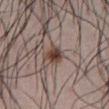workup: no biopsy performed (imaged during a skin exam)
patient: male, approximately 65 years of age
illumination: white-light
TBP lesion metrics: a footprint of about 4.5 mm² and a shape-asymmetry score of about 0.25 (0 = symmetric); an automated nevus-likeness rating near 95 out of 100 and a detector confidence of about 100 out of 100 that the crop contains a lesion
lesion diameter: about 2.5 mm
body site: the abdomen
imaging modality: ~15 mm crop, total-body skin-cancer survey